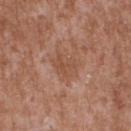<record>
  <image>
    <source>total-body photography crop</source>
    <field_of_view_mm>15</field_of_view_mm>
  </image>
  <lesion_size>
    <long_diameter_mm_approx>2.5</long_diameter_mm_approx>
  </lesion_size>
  <patient>
    <sex>male</sex>
    <age_approx>45</age_approx>
  </patient>
  <site>upper back</site>
  <lighting>white-light</lighting>
  <automated_metrics>
    <shape_asymmetry>0.4</shape_asymmetry>
    <cielab_L>50</cielab_L>
    <cielab_a>22</cielab_a>
    <cielab_b>31</cielab_b>
    <vs_skin_darker_L>6.0</vs_skin_darker_L>
    <vs_skin_contrast_norm>5.0</vs_skin_contrast_norm>
    <lesion_detection_confidence_0_100>100</lesion_detection_confidence_0_100>
  </automated_metrics>
</record>